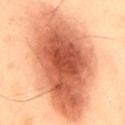Clinical impression: Part of a total-body skin-imaging series; this lesion was reviewed on a skin check and was not flagged for biopsy. Clinical summary: A close-up tile cropped from a whole-body skin photograph, about 15 mm across. A male subject aged 53 to 57. Automated tile analysis of the lesion measured a classifier nevus-likeness of about 100/100 and a detector confidence of about 100 out of 100 that the crop contains a lesion. Located on the back. This is a cross-polarized tile.